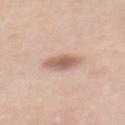Impression: Captured during whole-body skin photography for melanoma surveillance; the lesion was not biopsied. Clinical summary: Located on the mid back. A 15 mm crop from a total-body photograph taken for skin-cancer surveillance. A female subject approximately 40 years of age.